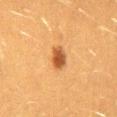Q: Was a biopsy performed?
A: catalogued during a skin exam; not biopsied
Q: What is the lesion's diameter?
A: ~3 mm (longest diameter)
Q: Lesion location?
A: the lower back
Q: Illumination type?
A: cross-polarized illumination
Q: Who is the patient?
A: female, aged 38 to 42
Q: How was this image acquired?
A: ~15 mm tile from a whole-body skin photo